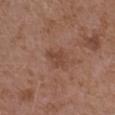Recorded during total-body skin imaging; not selected for excision or biopsy. A female patient, aged 33 to 37. The tile uses white-light illumination. Located on the left upper arm. A roughly 15 mm field-of-view crop from a total-body skin photograph.